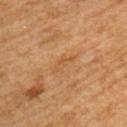A region of skin cropped from a whole-body photographic capture, roughly 15 mm wide.
The subject is a female aged 53 to 57.
The lesion is located on the left upper arm.